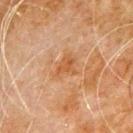Clinical impression:
Captured during whole-body skin photography for melanoma surveillance; the lesion was not biopsied.
Acquisition and patient details:
A male subject aged 78 to 82. From the left upper arm. Imaged with cross-polarized lighting. A roughly 15 mm field-of-view crop from a total-body skin photograph. The total-body-photography lesion software estimated a mean CIELAB color near L≈44 a*≈19 b*≈32 and roughly 6 lightness units darker than nearby skin. And it measured an automated nevus-likeness rating near 0 out of 100 and a lesion-detection confidence of about 100/100. Approximately 3.5 mm at its widest.